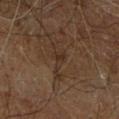Assessment:
The lesion was tiled from a total-body skin photograph and was not biopsied.
Context:
Longest diameter approximately 2.5 mm. The lesion is on the right leg. The tile uses cross-polarized illumination. This image is a 15 mm lesion crop taken from a total-body photograph. An algorithmic analysis of the crop reported a border-irregularity rating of about 5.5/10, a within-lesion color-variation index near 1/10, and peripheral color asymmetry of about 0. A male patient about 60 years old.